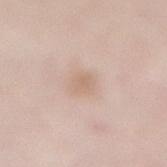{
  "biopsy_status": "not biopsied; imaged during a skin examination",
  "patient": {
    "sex": "female",
    "age_approx": 50
  },
  "site": "lower back",
  "image": {
    "source": "total-body photography crop",
    "field_of_view_mm": 15
  },
  "automated_metrics": {
    "area_mm2_approx": 3.5,
    "eccentricity": 0.8,
    "shape_asymmetry": 0.15,
    "peripheral_color_asymmetry": 0.5
  }
}